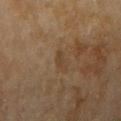Assessment: Captured during whole-body skin photography for melanoma surveillance; the lesion was not biopsied. Context: The subject is a female roughly 80 years of age. A close-up tile cropped from a whole-body skin photograph, about 15 mm across. This is a cross-polarized tile. Located on the right upper arm.